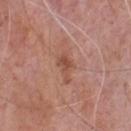Captured during whole-body skin photography for melanoma surveillance; the lesion was not biopsied.